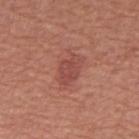| feature | finding |
|---|---|
| workup | no biopsy performed (imaged during a skin exam) |
| site | the abdomen |
| patient | male, aged approximately 65 |
| lighting | white-light |
| size | about 3.5 mm |
| image source | total-body-photography crop, ~15 mm field of view |
| image-analysis metrics | an area of roughly 7 mm² and a shape-asymmetry score of about 0.2 (0 = symmetric); a lesion color around L≈48 a*≈28 b*≈26 in CIELAB and a normalized border contrast of about 6; a border-irregularity rating of about 2/10, a within-lesion color-variation index near 2/10, and radial color variation of about 1; a lesion-detection confidence of about 100/100 |